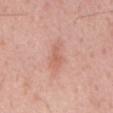The lesion was photographed on a routine skin check and not biopsied; there is no pathology result.
On the mid back.
A male subject approximately 55 years of age.
A 15 mm crop from a total-body photograph taken for skin-cancer surveillance.